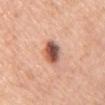follow-up: no biopsy performed (imaged during a skin exam)
automated metrics: an average lesion color of about L≈54 a*≈25 b*≈29 (CIELAB) and a lesion–skin lightness drop of about 19; a border-irregularity index near 1.5/10 and internal color variation of about 9.5 on a 0–10 scale; an automated nevus-likeness rating near 95 out of 100 and lesion-presence confidence of about 100/100
image: total-body-photography crop, ~15 mm field of view
diameter: ≈3.5 mm
lighting: white-light
subject: female, aged around 65
body site: the chest